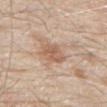Captured during whole-body skin photography for melanoma surveillance; the lesion was not biopsied. A male patient, approximately 80 years of age. This image is a 15 mm lesion crop taken from a total-body photograph. The lesion is located on the abdomen.